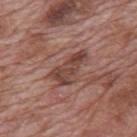– patient — male, aged approximately 70
– site — the mid back
– acquisition — total-body-photography crop, ~15 mm field of view
– image-analysis metrics — a lesion area of about 7.5 mm², an outline eccentricity of about 0.9 (0 = round, 1 = elongated), and a symmetry-axis asymmetry near 0.45; a border-irregularity index near 5/10, internal color variation of about 3 on a 0–10 scale, and a peripheral color-asymmetry measure near 1
– lighting — white-light illumination